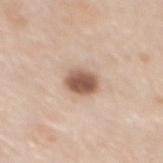Q: Who is the patient?
A: female, in their 50s
Q: How was this image acquired?
A: 15 mm crop, total-body photography
Q: Lesion location?
A: the upper back
Q: How large is the lesion?
A: ≈3 mm
Q: What did automated image analysis measure?
A: a normalized border contrast of about 10.5; a border-irregularity index near 1.5/10, internal color variation of about 4.5 on a 0–10 scale, and radial color variation of about 1.5; a detector confidence of about 100 out of 100 that the crop contains a lesion
Q: Illumination type?
A: white-light illumination